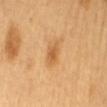notes: imaged on a skin check; not biopsied | lighting: cross-polarized | imaging modality: 15 mm crop, total-body photography | lesion diameter: ~3 mm (longest diameter) | subject: female, aged 58 to 62 | anatomic site: the mid back.